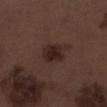<record>
<lighting>white-light</lighting>
<patient>
  <sex>male</sex>
  <age_approx>70</age_approx>
</patient>
<automated_metrics>
  <cielab_L>24</cielab_L>
  <cielab_a>15</cielab_a>
  <cielab_b>18</cielab_b>
  <vs_skin_contrast_norm>9.0</vs_skin_contrast_norm>
  <color_variation_0_10>3.0</color_variation_0_10>
  <peripheral_color_asymmetry>1.0</peripheral_color_asymmetry>
  <nevus_likeness_0_100>80</nevus_likeness_0_100>
</automated_metrics>
<site>right lower leg</site>
<lesion_size>
  <long_diameter_mm_approx>3.5</long_diameter_mm_approx>
</lesion_size>
<image>
  <source>total-body photography crop</source>
  <field_of_view_mm>15</field_of_view_mm>
</image>
</record>